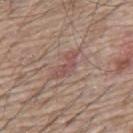Clinical impression:
Captured during whole-body skin photography for melanoma surveillance; the lesion was not biopsied.
Background:
A 15 mm crop from a total-body photograph taken for skin-cancer surveillance. An algorithmic analysis of the crop reported a nevus-likeness score of about 0/100 and a detector confidence of about 65 out of 100 that the crop contains a lesion. Imaged with white-light lighting. On the upper back. A male subject in their mid-60s. About 4.5 mm across.